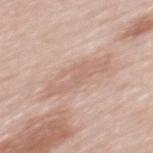Image and clinical context: Automated tile analysis of the lesion measured a classifier nevus-likeness of about 0/100 and lesion-presence confidence of about 90/100. A female subject roughly 60 years of age. The tile uses white-light illumination. A 15 mm crop from a total-body photograph taken for skin-cancer surveillance. From the upper back.